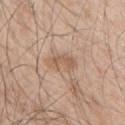Part of a total-body skin-imaging series; this lesion was reviewed on a skin check and was not flagged for biopsy. From the right upper arm. Approximately 4 mm at its widest. The patient is a male aged around 50. A lesion tile, about 15 mm wide, cut from a 3D total-body photograph.